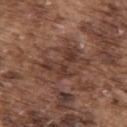Impression:
Recorded during total-body skin imaging; not selected for excision or biopsy.